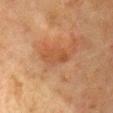Q: Where on the body is the lesion?
A: the chest
Q: How was the tile lit?
A: cross-polarized illumination
Q: What kind of image is this?
A: 15 mm crop, total-body photography
Q: What are the patient's age and sex?
A: female, approximately 50 years of age